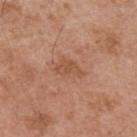illumination=white-light
lesion size=about 3.5 mm
acquisition=~15 mm crop, total-body skin-cancer survey
site=the upper back
TBP lesion metrics=a footprint of about 5.5 mm², a shape eccentricity near 0.8, and two-axis asymmetry of about 0.4; a mean CIELAB color near L≈52 a*≈23 b*≈33, roughly 7 lightness units darker than nearby skin, and a normalized lesion–skin contrast near 5.5; a border-irregularity index near 4/10 and a color-variation rating of about 1.5/10; a classifier nevus-likeness of about 0/100 and a detector confidence of about 100 out of 100 that the crop contains a lesion
patient=male, roughly 55 years of age